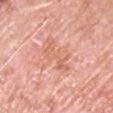notes=no biopsy performed (imaged during a skin exam) | image-analysis metrics=a shape eccentricity near 0.8; border irregularity of about 7.5 on a 0–10 scale and a within-lesion color-variation index near 2/10 | patient=male, aged around 80 | site=the chest | image=~15 mm crop, total-body skin-cancer survey.